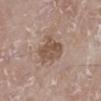Q: Was a biopsy performed?
A: catalogued during a skin exam; not biopsied
Q: How was this image acquired?
A: ~15 mm tile from a whole-body skin photo
Q: What are the patient's age and sex?
A: male, aged 78 to 82
Q: Where on the body is the lesion?
A: the leg
Q: What is the lesion's diameter?
A: ~4 mm (longest diameter)
Q: What did automated image analysis measure?
A: a lesion area of about 10 mm², an eccentricity of roughly 0.7, and two-axis asymmetry of about 0.3; a within-lesion color-variation index near 3.5/10 and radial color variation of about 1.5; a classifier nevus-likeness of about 5/100 and a detector confidence of about 100 out of 100 that the crop contains a lesion
Q: How was the tile lit?
A: white-light illumination